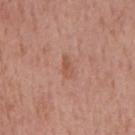The lesion was photographed on a routine skin check and not biopsied; there is no pathology result.
Longest diameter approximately 2.5 mm.
A region of skin cropped from a whole-body photographic capture, roughly 15 mm wide.
A male subject, aged around 55.
The lesion is located on the mid back.
The tile uses white-light illumination.